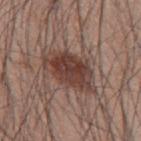workup = imaged on a skin check; not biopsied
site = the back
diameter = ~6.5 mm (longest diameter)
illumination = white-light illumination
patient = male, roughly 60 years of age
imaging modality = ~15 mm tile from a whole-body skin photo